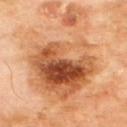<tbp_lesion>
  <biopsy_status>not biopsied; imaged during a skin examination</biopsy_status>
  <patient>
    <sex>male</sex>
    <age_approx>70</age_approx>
  </patient>
  <lighting>cross-polarized</lighting>
  <site>back</site>
  <image>
    <source>total-body photography crop</source>
    <field_of_view_mm>15</field_of_view_mm>
  </image>
  <automated_metrics>
    <area_mm2_approx>65.0</area_mm2_approx>
    <eccentricity>0.8</eccentricity>
    <shape_asymmetry>0.4</shape_asymmetry>
    <nevus_likeness_0_100>90</nevus_likeness_0_100>
    <lesion_detection_confidence_0_100>100</lesion_detection_confidence_0_100>
  </automated_metrics>
  <lesion_size>
    <long_diameter_mm_approx>14.0</long_diameter_mm_approx>
  </lesion_size>
</tbp_lesion>